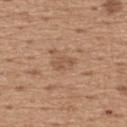Case summary:
– follow-up · total-body-photography surveillance lesion; no biopsy
– illumination · white-light illumination
– image-analysis metrics · a mean CIELAB color near L≈53 a*≈19 b*≈31, about 8 CIELAB-L* units darker than the surrounding skin, and a lesion-to-skin contrast of about 5.5 (normalized; higher = more distinct); a border-irregularity index near 5.5/10 and a within-lesion color-variation index near 0/10; a nevus-likeness score of about 0/100 and a lesion-detection confidence of about 100/100
– location · the upper back
– subject · male, aged approximately 65
– lesion diameter · about 2.5 mm
– acquisition · 15 mm crop, total-body photography